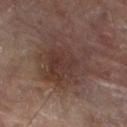Clinical impression:
The lesion was photographed on a routine skin check and not biopsied; there is no pathology result.
Clinical summary:
Located on the right leg. The total-body-photography lesion software estimated a footprint of about 27 mm², an eccentricity of roughly 0.65, and two-axis asymmetry of about 0.3. It also reported a mean CIELAB color near L≈32 a*≈15 b*≈19 and a lesion–skin lightness drop of about 6. A 15 mm close-up extracted from a 3D total-body photography capture. The recorded lesion diameter is about 7.5 mm. A female patient roughly 80 years of age.